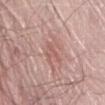Clinical impression: Part of a total-body skin-imaging series; this lesion was reviewed on a skin check and was not flagged for biopsy. Background: Cropped from a total-body skin-imaging series; the visible field is about 15 mm. From the abdomen. Captured under white-light illumination. The patient is a male in their 80s. Longest diameter approximately 3 mm.